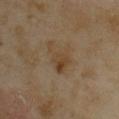{
  "site": "upper back",
  "patient": {
    "sex": "female",
    "age_approx": 35
  },
  "automated_metrics": {
    "border_irregularity_0_10": 7.0,
    "color_variation_0_10": 4.5,
    "peripheral_color_asymmetry": 1.5
  },
  "image": {
    "source": "total-body photography crop",
    "field_of_view_mm": 15
  },
  "lesion_size": {
    "long_diameter_mm_approx": 4.0
  }
}